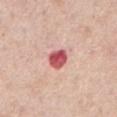This lesion was catalogued during total-body skin photography and was not selected for biopsy. On the abdomen. The patient is a male aged 73 to 77. This is a white-light tile. Measured at roughly 3 mm in maximum diameter. A lesion tile, about 15 mm wide, cut from a 3D total-body photograph.